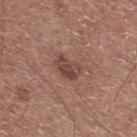{"biopsy_status": "not biopsied; imaged during a skin examination", "lesion_size": {"long_diameter_mm_approx": 3.0}, "image": {"source": "total-body photography crop", "field_of_view_mm": 15}, "site": "upper back", "patient": {"sex": "male", "age_approx": 60}}